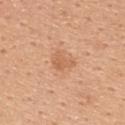{
  "biopsy_status": "not biopsied; imaged during a skin examination",
  "site": "upper back",
  "patient": {
    "sex": "female",
    "age_approx": 45
  },
  "image": {
    "source": "total-body photography crop",
    "field_of_view_mm": 15
  }
}